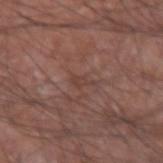Clinical impression:
Recorded during total-body skin imaging; not selected for excision or biopsy.
Acquisition and patient details:
About 2.5 mm across. Cropped from a total-body skin-imaging series; the visible field is about 15 mm. An algorithmic analysis of the crop reported a shape-asymmetry score of about 0.45 (0 = symmetric). It also reported border irregularity of about 5.5 on a 0–10 scale and radial color variation of about 0. It also reported an automated nevus-likeness rating near 0 out of 100. On the arm. A male subject, in their mid- to late 70s. This is a white-light tile.